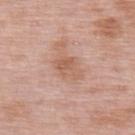| feature | finding |
|---|---|
| biopsy status | no biopsy performed (imaged during a skin exam) |
| size | ≈3.5 mm |
| image-analysis metrics | a border-irregularity rating of about 3/10, a within-lesion color-variation index near 3.5/10, and a peripheral color-asymmetry measure near 1; lesion-presence confidence of about 100/100 |
| image | total-body-photography crop, ~15 mm field of view |
| location | the upper back |
| patient | female, in their 50s |
| tile lighting | white-light illumination |